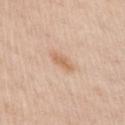Recorded during total-body skin imaging; not selected for excision or biopsy.
Located on the chest.
A 15 mm crop from a total-body photograph taken for skin-cancer surveillance.
Imaged with white-light lighting.
The subject is a male aged approximately 60.
The lesion's longest dimension is about 3 mm.
The lesion-visualizer software estimated a lesion area of about 3 mm², a shape eccentricity near 0.9, and a shape-asymmetry score of about 0.3 (0 = symmetric). The analysis additionally found a lesion color around L≈64 a*≈20 b*≈34 in CIELAB, a lesion–skin lightness drop of about 9, and a lesion-to-skin contrast of about 6.5 (normalized; higher = more distinct). The analysis additionally found a border-irregularity rating of about 3/10, a within-lesion color-variation index near 1/10, and a peripheral color-asymmetry measure near 0.5. The analysis additionally found a nevus-likeness score of about 45/100.